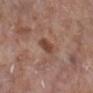notes: imaged on a skin check; not biopsied
patient: female, about 75 years old
body site: the left lower leg
image: ~15 mm tile from a whole-body skin photo
diameter: about 2.5 mm
lighting: white-light illumination